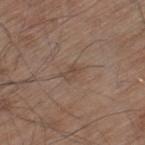Located on the leg. About 2.5 mm across. A region of skin cropped from a whole-body photographic capture, roughly 15 mm wide. A male patient, in their 80s. Imaged with white-light lighting.